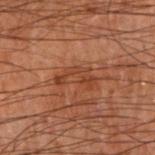Imaged during a routine full-body skin examination; the lesion was not biopsied and no histopathology is available. This is a cross-polarized tile. Cropped from a whole-body photographic skin survey; the tile spans about 15 mm. Approximately 4.5 mm at its widest. Located on the arm. A male subject about 70 years old.